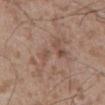Imaged during a routine full-body skin examination; the lesion was not biopsied and no histopathology is available.
A 15 mm close-up tile from a total-body photography series done for melanoma screening.
Longest diameter approximately 8.5 mm.
The patient is a male aged 53–57.
The lesion is located on the abdomen.
Captured under white-light illumination.